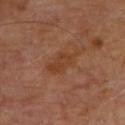  biopsy_status: not biopsied; imaged during a skin examination
  lighting: cross-polarized
  site: back
  image:
    source: total-body photography crop
    field_of_view_mm: 15
  patient:
    sex: male
    age_approx: 70